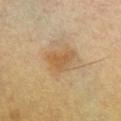biopsy status = total-body-photography surveillance lesion; no biopsy
tile lighting = cross-polarized illumination
image = ~15 mm crop, total-body skin-cancer survey
site = the front of the torso
size = ≈4 mm
patient = male, aged approximately 60